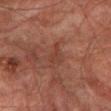Findings:
* biopsy status: total-body-photography surveillance lesion; no biopsy
* illumination: cross-polarized
* site: the left lower leg
* TBP lesion metrics: a lesion area of about 3.5 mm², an outline eccentricity of about 0.9 (0 = round, 1 = elongated), and a symmetry-axis asymmetry near 0.5; a mean CIELAB color near L≈32 a*≈20 b*≈24, about 5 CIELAB-L* units darker than the surrounding skin, and a normalized border contrast of about 5; a nevus-likeness score of about 0/100
* patient: male, aged around 75
* size: about 3.5 mm
* acquisition: ~15 mm tile from a whole-body skin photo